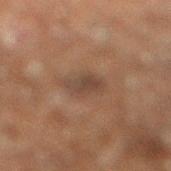This lesion was catalogued during total-body skin photography and was not selected for biopsy. The patient is a male roughly 60 years of age. The lesion is located on the right lower leg. A close-up tile cropped from a whole-body skin photograph, about 15 mm across.